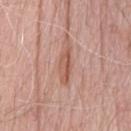<case>
<patient>
  <sex>male</sex>
  <age_approx>75</age_approx>
</patient>
<lesion_size>
  <long_diameter_mm_approx>4.5</long_diameter_mm_approx>
</lesion_size>
<image>
  <source>total-body photography crop</source>
  <field_of_view_mm>15</field_of_view_mm>
</image>
<lighting>white-light</lighting>
<site>chest</site>
</case>